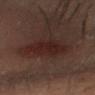The lesion is on the head or neck.
Automated tile analysis of the lesion measured a mean CIELAB color near L≈17 a*≈15 b*≈16, a lesion–skin lightness drop of about 5, and a lesion-to-skin contrast of about 7.5 (normalized; higher = more distinct). It also reported border irregularity of about 3 on a 0–10 scale, internal color variation of about 2.5 on a 0–10 scale, and radial color variation of about 1.
About 4.5 mm across.
A lesion tile, about 15 mm wide, cut from a 3D total-body photograph.
Captured under cross-polarized illumination.
A male patient aged 28 to 32.